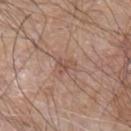Assessment:
The lesion was photographed on a routine skin check and not biopsied; there is no pathology result.
Acquisition and patient details:
The subject is a male in their 80s. Cropped from a total-body skin-imaging series; the visible field is about 15 mm. Located on the left arm. Automated image analysis of the tile measured a border-irregularity rating of about 3/10, a within-lesion color-variation index near 3/10, and a peripheral color-asymmetry measure near 1. The analysis additionally found a nevus-likeness score of about 0/100.